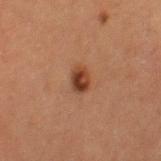Clinical impression: This lesion was catalogued during total-body skin photography and was not selected for biopsy. Acquisition and patient details: The patient is a female aged approximately 50. On the right thigh. Cropped from a total-body skin-imaging series; the visible field is about 15 mm.